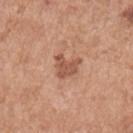Captured during whole-body skin photography for melanoma surveillance; the lesion was not biopsied.
Automated tile analysis of the lesion measured a footprint of about 5.5 mm² and a shape eccentricity near 0.4. It also reported an average lesion color of about L≈54 a*≈24 b*≈31 (CIELAB). The analysis additionally found border irregularity of about 5.5 on a 0–10 scale, a color-variation rating of about 2/10, and radial color variation of about 1.
A male subject, in their mid-50s.
On the chest.
The lesion's longest dimension is about 3 mm.
A roughly 15 mm field-of-view crop from a total-body skin photograph.
Imaged with white-light lighting.